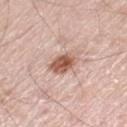{"patient": {"sex": "male", "age_approx": 80}, "lighting": "white-light", "image": {"source": "total-body photography crop", "field_of_view_mm": 15}, "lesion_size": {"long_diameter_mm_approx": 3.0}, "site": "left thigh"}